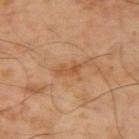• notes: no biopsy performed (imaged during a skin exam)
• illumination: cross-polarized illumination
• site: the left arm
• size: ~3 mm (longest diameter)
• patient: male, in their 50s
• image-analysis metrics: a lesion color around L≈52 a*≈22 b*≈37 in CIELAB, roughly 7 lightness units darker than nearby skin, and a normalized lesion–skin contrast near 6; a border-irregularity index near 4/10, a within-lesion color-variation index near 0/10, and peripheral color asymmetry of about 0; a nevus-likeness score of about 0/100 and a lesion-detection confidence of about 100/100
• imaging modality: ~15 mm crop, total-body skin-cancer survey